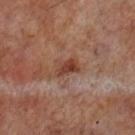biopsy status=no biopsy performed (imaged during a skin exam)
automated metrics=a lesion color around L≈40 a*≈22 b*≈28 in CIELAB, roughly 10 lightness units darker than nearby skin, and a normalized lesion–skin contrast near 8.5; internal color variation of about 3.5 on a 0–10 scale and a peripheral color-asymmetry measure near 1.5; a nevus-likeness score of about 0/100 and a lesion-detection confidence of about 100/100
body site=the left lower leg
acquisition=total-body-photography crop, ~15 mm field of view
tile lighting=cross-polarized illumination
patient=male, aged around 70
lesion diameter=≈3 mm